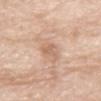Impression: The lesion was tiled from a total-body skin photograph and was not biopsied. Acquisition and patient details: A 15 mm crop from a total-body photograph taken for skin-cancer surveillance. Imaged with white-light lighting. On the front of the torso. A male patient, roughly 80 years of age. An algorithmic analysis of the crop reported a lesion-to-skin contrast of about 5 (normalized; higher = more distinct). It also reported a border-irregularity rating of about 2.5/10, internal color variation of about 2 on a 0–10 scale, and peripheral color asymmetry of about 0.5.